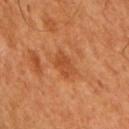<lesion>
  <biopsy_status>not biopsied; imaged during a skin examination</biopsy_status>
  <site>right upper arm</site>
  <patient>
    <sex>male</sex>
    <age_approx>50</age_approx>
  </patient>
  <image>
    <source>total-body photography crop</source>
    <field_of_view_mm>15</field_of_view_mm>
  </image>
</lesion>